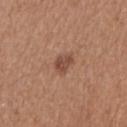Assessment: The lesion was tiled from a total-body skin photograph and was not biopsied. Clinical summary: A female subject aged approximately 30. A region of skin cropped from a whole-body photographic capture, roughly 15 mm wide. Imaged with white-light lighting. On the right forearm. About 2.5 mm across. An algorithmic analysis of the crop reported an area of roughly 4.5 mm², an eccentricity of roughly 0.7, and two-axis asymmetry of about 0.3. And it measured a lesion color around L≈48 a*≈22 b*≈29 in CIELAB. And it measured a classifier nevus-likeness of about 80/100.